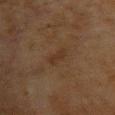Part of a total-body skin-imaging series; this lesion was reviewed on a skin check and was not flagged for biopsy. A 15 mm close-up tile from a total-body photography series done for melanoma screening. This is a cross-polarized tile. A female patient roughly 80 years of age. On the upper back.